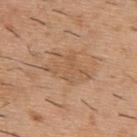Background:
Located on the upper back. A male patient, roughly 40 years of age. Approximately 5 mm at its widest. Cropped from a total-body skin-imaging series; the visible field is about 15 mm.